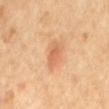Part of a total-body skin-imaging series; this lesion was reviewed on a skin check and was not flagged for biopsy. The lesion is on the back. A female subject, approximately 65 years of age. Automated image analysis of the tile measured border irregularity of about 3 on a 0–10 scale and a within-lesion color-variation index near 2.5/10. A lesion tile, about 15 mm wide, cut from a 3D total-body photograph. Longest diameter approximately 4 mm.